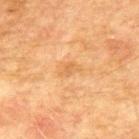workup: total-body-photography surveillance lesion; no biopsy
site: the back
tile lighting: cross-polarized illumination
image: 15 mm crop, total-body photography
subject: male, roughly 75 years of age